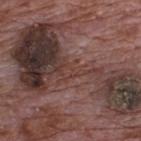Part of a total-body skin-imaging series; this lesion was reviewed on a skin check and was not flagged for biopsy.